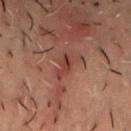<record>
  <biopsy_status>not biopsied; imaged during a skin examination</biopsy_status>
  <site>chest</site>
  <lighting>cross-polarized</lighting>
  <image>
    <source>total-body photography crop</source>
    <field_of_view_mm>15</field_of_view_mm>
  </image>
  <patient>
    <sex>male</sex>
    <age_approx>50</age_approx>
  </patient>
</record>